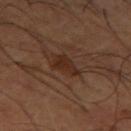A 15 mm crop from a total-body photograph taken for skin-cancer surveillance.
Longest diameter approximately 3.5 mm.
The tile uses cross-polarized illumination.
The lesion is on the left thigh.
A male subject, aged 63–67.
Automated tile analysis of the lesion measured a border-irregularity rating of about 4/10, internal color variation of about 2 on a 0–10 scale, and peripheral color asymmetry of about 1.